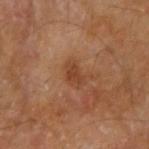<tbp_lesion>
<biopsy_status>not biopsied; imaged during a skin examination</biopsy_status>
<patient>
  <sex>male</sex>
  <age_approx>70</age_approx>
</patient>
<site>arm</site>
<image>
  <source>total-body photography crop</source>
  <field_of_view_mm>15</field_of_view_mm>
</image>
</tbp_lesion>